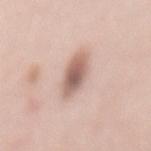Part of a total-body skin-imaging series; this lesion was reviewed on a skin check and was not flagged for biopsy. The lesion is located on the mid back. A female subject about 40 years old. Imaged with white-light lighting. The lesion's longest dimension is about 5 mm. An algorithmic analysis of the crop reported an eccentricity of roughly 0.9 and a symmetry-axis asymmetry near 0.3. A 15 mm close-up extracted from a 3D total-body photography capture.